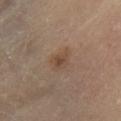Recorded during total-body skin imaging; not selected for excision or biopsy. The lesion is on the leg. A region of skin cropped from a whole-body photographic capture, roughly 15 mm wide. This is a cross-polarized tile. A female patient aged approximately 60.